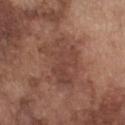Captured during whole-body skin photography for melanoma surveillance; the lesion was not biopsied.
The total-body-photography lesion software estimated a lesion area of about 21 mm², a shape eccentricity near 0.8, and a shape-asymmetry score of about 0.25 (0 = symmetric).
Longest diameter approximately 6.5 mm.
Imaged with white-light lighting.
A male patient aged 73–77.
From the chest.
Cropped from a whole-body photographic skin survey; the tile spans about 15 mm.